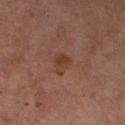Assessment: Part of a total-body skin-imaging series; this lesion was reviewed on a skin check and was not flagged for biopsy. Image and clinical context: A female patient, aged 58–62. Approximately 3 mm at its widest. Located on the leg. This is a cross-polarized tile. This image is a 15 mm lesion crop taken from a total-body photograph.